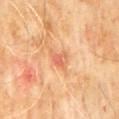Clinical impression: This lesion was catalogued during total-body skin photography and was not selected for biopsy. Context: The lesion-visualizer software estimated a mean CIELAB color near L≈63 a*≈28 b*≈37, about 9 CIELAB-L* units darker than the surrounding skin, and a normalized lesion–skin contrast near 5.5. Captured under cross-polarized illumination. Cropped from a total-body skin-imaging series; the visible field is about 15 mm. The patient is a male about 60 years old. The lesion is located on the abdomen. Longest diameter approximately 2.5 mm.